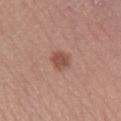<case>
<biopsy_status>not biopsied; imaged during a skin examination</biopsy_status>
<site>right lower leg</site>
<lighting>white-light</lighting>
<image>
  <source>total-body photography crop</source>
  <field_of_view_mm>15</field_of_view_mm>
</image>
<patient>
  <sex>female</sex>
  <age_approx>40</age_approx>
</patient>
</case>